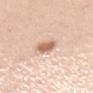{
  "biopsy_status": "not biopsied; imaged during a skin examination",
  "patient": {
    "sex": "female",
    "age_approx": 40
  },
  "site": "mid back",
  "image": {
    "source": "total-body photography crop",
    "field_of_view_mm": 15
  }
}